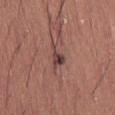follow-up = catalogued during a skin exam; not biopsied
acquisition = total-body-photography crop, ~15 mm field of view
patient = male, aged 43 to 47
body site = the right thigh